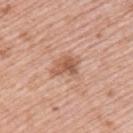| field | value |
|---|---|
| notes | no biopsy performed (imaged during a skin exam) |
| body site | the back |
| lighting | white-light |
| subject | male, aged around 55 |
| acquisition | ~15 mm crop, total-body skin-cancer survey |
| lesion diameter | ~3.5 mm (longest diameter) |